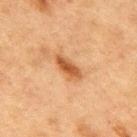Image and clinical context:
The lesion is on the back. An algorithmic analysis of the crop reported an average lesion color of about L≈45 a*≈22 b*≈35 (CIELAB), roughly 11 lightness units darker than nearby skin, and a normalized lesion–skin contrast near 9. The patient is a male aged around 75. A 15 mm close-up extracted from a 3D total-body photography capture. The tile uses cross-polarized illumination. Measured at roughly 4 mm in maximum diameter.